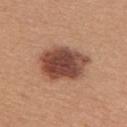The lesion is located on the upper back.
Imaged with white-light lighting.
The patient is a female about 45 years old.
About 6 mm across.
A lesion tile, about 15 mm wide, cut from a 3D total-body photograph.
Automated tile analysis of the lesion measured an average lesion color of about L≈46 a*≈23 b*≈28 (CIELAB), a lesion–skin lightness drop of about 17, and a normalized lesion–skin contrast near 12.